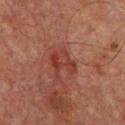{
  "biopsy_status": "not biopsied; imaged during a skin examination",
  "automated_metrics": {
    "cielab_L": 30,
    "cielab_a": 24,
    "cielab_b": 24,
    "vs_skin_darker_L": 6.0,
    "vs_skin_contrast_norm": 6.5,
    "color_variation_0_10": 4.0,
    "peripheral_color_asymmetry": 1.0
  },
  "patient": {
    "sex": "male",
    "age_approx": 65
  },
  "site": "upper back",
  "lesion_size": {
    "long_diameter_mm_approx": 3.5
  },
  "lighting": "cross-polarized",
  "image": {
    "source": "total-body photography crop",
    "field_of_view_mm": 15
  }
}